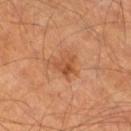Assessment:
Recorded during total-body skin imaging; not selected for excision or biopsy.
Image and clinical context:
From the right thigh. This is a cross-polarized tile. Measured at roughly 2.5 mm in maximum diameter. This image is a 15 mm lesion crop taken from a total-body photograph. A male patient, in their mid- to late 60s. The lesion-visualizer software estimated a lesion area of about 4 mm², a shape eccentricity near 0.5, and two-axis asymmetry of about 0.45. The software also gave a mean CIELAB color near L≈45 a*≈24 b*≈34 and roughly 8 lightness units darker than nearby skin. It also reported a within-lesion color-variation index near 3.5/10 and radial color variation of about 1. The analysis additionally found a nevus-likeness score of about 30/100.